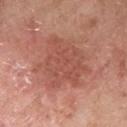follow-up: no biopsy performed (imaged during a skin exam) | image source: ~15 mm crop, total-body skin-cancer survey | automated lesion analysis: an outline eccentricity of about 0.5 (0 = round, 1 = elongated) and a shape-asymmetry score of about 0.3 (0 = symmetric); an average lesion color of about L≈52 a*≈27 b*≈28 (CIELAB), about 8 CIELAB-L* units darker than the surrounding skin, and a normalized lesion–skin contrast near 5.5; an automated nevus-likeness rating near 0 out of 100 and a lesion-detection confidence of about 100/100 | lesion diameter: ~6.5 mm (longest diameter) | body site: the right forearm | lighting: white-light | patient: female, roughly 70 years of age.